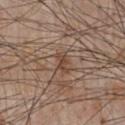Captured during whole-body skin photography for melanoma surveillance; the lesion was not biopsied. Cropped from a whole-body photographic skin survey; the tile spans about 15 mm. Located on the chest. Approximately 2.5 mm at its widest. The subject is a male aged 58 to 62.